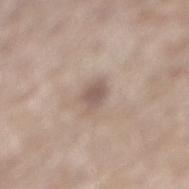{"lighting": "white-light", "image": {"source": "total-body photography crop", "field_of_view_mm": 15}, "patient": {"sex": "male", "age_approx": 70}, "site": "right lower leg", "lesion_size": {"long_diameter_mm_approx": 2.5}}